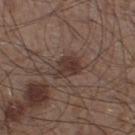  biopsy_status: not biopsied; imaged during a skin examination
  lighting: white-light
  patient:
    sex: male
    age_approx: 60
  lesion_size:
    long_diameter_mm_approx: 3.5
  automated_metrics:
    border_irregularity_0_10: 2.5
    color_variation_0_10: 3.0
    peripheral_color_asymmetry: 1.0
  image:
    source: total-body photography crop
    field_of_view_mm: 15
  site: left lower leg